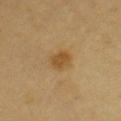biopsy status: catalogued during a skin exam; not biopsied
patient: male, approximately 60 years of age
imaging modality: total-body-photography crop, ~15 mm field of view
tile lighting: cross-polarized
anatomic site: the chest